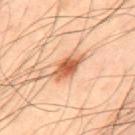Part of a total-body skin-imaging series; this lesion was reviewed on a skin check and was not flagged for biopsy.
Imaged with cross-polarized lighting.
Longest diameter approximately 5 mm.
A male patient in their 50s.
Automated tile analysis of the lesion measured a color-variation rating of about 4.5/10 and a peripheral color-asymmetry measure near 1.5. And it measured an automated nevus-likeness rating near 95 out of 100 and lesion-presence confidence of about 100/100.
The lesion is on the upper back.
A 15 mm close-up tile from a total-body photography series done for melanoma screening.